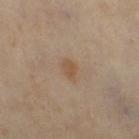<case>
  <biopsy_status>not biopsied; imaged during a skin examination</biopsy_status>
  <lesion_size>
    <long_diameter_mm_approx>2.5</long_diameter_mm_approx>
  </lesion_size>
  <lighting>cross-polarized</lighting>
  <site>right thigh</site>
  <automated_metrics>
    <eccentricity>0.8</eccentricity>
    <shape_asymmetry>0.25</shape_asymmetry>
    <cielab_L>49</cielab_L>
    <cielab_a>15</cielab_a>
    <cielab_b>30</cielab_b>
    <vs_skin_contrast_norm>6.5</vs_skin_contrast_norm>
  </automated_metrics>
  <patient>
    <sex>female</sex>
    <age_approx>50</age_approx>
  </patient>
  <image>
    <source>total-body photography crop</source>
    <field_of_view_mm>15</field_of_view_mm>
  </image>
</case>